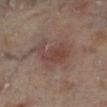Q: Is there a histopathology result?
A: no biopsy performed (imaged during a skin exam)
Q: How was this image acquired?
A: ~15 mm crop, total-body skin-cancer survey
Q: What is the anatomic site?
A: the right lower leg
Q: How was the tile lit?
A: cross-polarized
Q: Patient demographics?
A: male, aged 63–67
Q: Lesion size?
A: ≈5 mm
Q: What did automated image analysis measure?
A: an outline eccentricity of about 0.7 (0 = round, 1 = elongated) and a shape-asymmetry score of about 0.45 (0 = symmetric); internal color variation of about 3.5 on a 0–10 scale and radial color variation of about 1; a nevus-likeness score of about 75/100 and a lesion-detection confidence of about 100/100